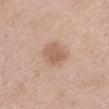The lesion was photographed on a routine skin check and not biopsied; there is no pathology result. The recorded lesion diameter is about 3.5 mm. Cropped from a total-body skin-imaging series; the visible field is about 15 mm. On the mid back. The tile uses white-light illumination. A female subject, in their 50s. Automated tile analysis of the lesion measured a lesion area of about 8.5 mm², an eccentricity of roughly 0.6, and a symmetry-axis asymmetry near 0.15. And it measured a classifier nevus-likeness of about 25/100 and a detector confidence of about 100 out of 100 that the crop contains a lesion.